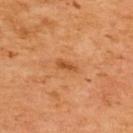This lesion was catalogued during total-body skin photography and was not selected for biopsy.
A lesion tile, about 15 mm wide, cut from a 3D total-body photograph.
About 3 mm across.
The patient is a male aged around 65.
Imaged with cross-polarized lighting.
Automated image analysis of the tile measured an area of roughly 3 mm², a shape eccentricity near 0.9, and two-axis asymmetry of about 0.25. The analysis additionally found border irregularity of about 2.5 on a 0–10 scale, internal color variation of about 1 on a 0–10 scale, and peripheral color asymmetry of about 0. It also reported a classifier nevus-likeness of about 0/100 and a detector confidence of about 100 out of 100 that the crop contains a lesion.